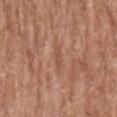The recorded lesion diameter is about 3 mm. The patient is a female aged around 80. On the arm. A 15 mm close-up tile from a total-body photography series done for melanoma screening.